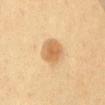Clinical impression:
Imaged during a routine full-body skin examination; the lesion was not biopsied and no histopathology is available.
Background:
The total-body-photography lesion software estimated a footprint of about 9.5 mm², an eccentricity of roughly 0.5, and two-axis asymmetry of about 0.15. It also reported roughly 10 lightness units darker than nearby skin and a normalized lesion–skin contrast near 7. It also reported a color-variation rating of about 3/10 and a peripheral color-asymmetry measure near 0.5. It also reported a nevus-likeness score of about 95/100 and a lesion-detection confidence of about 100/100. A female subject aged 53 to 57. Cropped from a whole-body photographic skin survey; the tile spans about 15 mm. The lesion is located on the chest. The tile uses cross-polarized illumination. Longest diameter approximately 3.5 mm.